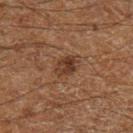<tbp_lesion>
<biopsy_status>not biopsied; imaged during a skin examination</biopsy_status>
<site>left lower leg</site>
<automated_metrics>
  <cielab_L>27</cielab_L>
  <cielab_a>16</cielab_a>
  <cielab_b>22</cielab_b>
  <vs_skin_darker_L>7.0</vs_skin_darker_L>
  <vs_skin_contrast_norm>8.0</vs_skin_contrast_norm>
  <border_irregularity_0_10>2.0</border_irregularity_0_10>
  <color_variation_0_10>4.5</color_variation_0_10>
  <peripheral_color_asymmetry>2.0</peripheral_color_asymmetry>
</automated_metrics>
<patient>
  <sex>male</sex>
  <age_approx>60</age_approx>
</patient>
<lesion_size>
  <long_diameter_mm_approx>3.0</long_diameter_mm_approx>
</lesion_size>
<image>
  <source>total-body photography crop</source>
  <field_of_view_mm>15</field_of_view_mm>
</image>
<lighting>cross-polarized</lighting>
</tbp_lesion>